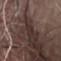* lighting · white-light
* imaging modality · 15 mm crop, total-body photography
* lesion diameter · ~3 mm (longest diameter)
* patient · male, aged 63–67
* site · the abdomen